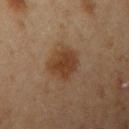Captured during whole-body skin photography for melanoma surveillance; the lesion was not biopsied. The lesion-visualizer software estimated a lesion color around L≈39 a*≈18 b*≈30 in CIELAB and a lesion-to-skin contrast of about 8 (normalized; higher = more distinct). It also reported border irregularity of about 2 on a 0–10 scale and a color-variation rating of about 2.5/10. The analysis additionally found a classifier nevus-likeness of about 90/100 and lesion-presence confidence of about 100/100. The lesion is on the arm. A region of skin cropped from a whole-body photographic capture, roughly 15 mm wide. Captured under cross-polarized illumination. A male patient, aged 53 to 57.